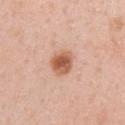Recorded during total-body skin imaging; not selected for excision or biopsy. The recorded lesion diameter is about 3.5 mm. Captured under white-light illumination. A region of skin cropped from a whole-body photographic capture, roughly 15 mm wide. On the right upper arm. The subject is a female aged approximately 35. The total-body-photography lesion software estimated an outline eccentricity of about 0.6 (0 = round, 1 = elongated) and two-axis asymmetry of about 0.15. The analysis additionally found a mean CIELAB color near L≈59 a*≈24 b*≈33, about 14 CIELAB-L* units darker than the surrounding skin, and a normalized lesion–skin contrast near 9.5. The analysis additionally found a nevus-likeness score of about 100/100 and a detector confidence of about 100 out of 100 that the crop contains a lesion.